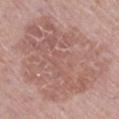{"biopsy_status": "not biopsied; imaged during a skin examination", "patient": {"sex": "female", "age_approx": 55}, "lesion_size": {"long_diameter_mm_approx": 12.5}, "image": {"source": "total-body photography crop", "field_of_view_mm": 15}, "lighting": "white-light", "automated_metrics": {"cielab_L": 58, "cielab_a": 20, "cielab_b": 24, "vs_skin_contrast_norm": 5.5, "lesion_detection_confidence_0_100": 100}, "site": "right lower leg"}